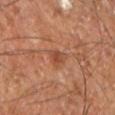  biopsy_status: not biopsied; imaged during a skin examination
  patient:
    sex: male
    age_approx: 60
  lighting: cross-polarized
  site: right leg
  lesion_size:
    long_diameter_mm_approx: 2.5
  image:
    source: total-body photography crop
    field_of_view_mm: 15